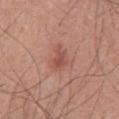<lesion>
  <biopsy_status>not biopsied; imaged during a skin examination</biopsy_status>
  <patient>
    <sex>male</sex>
    <age_approx>55</age_approx>
  </patient>
  <site>chest</site>
  <lighting>white-light</lighting>
  <image>
    <source>total-body photography crop</source>
    <field_of_view_mm>15</field_of_view_mm>
  </image>
</lesion>